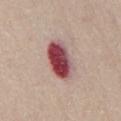Assessment:
This lesion was catalogued during total-body skin photography and was not selected for biopsy.
Image and clinical context:
The subject is a male aged approximately 65. This is a white-light tile. This image is a 15 mm lesion crop taken from a total-body photograph. About 5.5 mm across. From the chest.